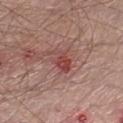Q: Was a biopsy performed?
A: total-body-photography surveillance lesion; no biopsy
Q: Automated lesion metrics?
A: a footprint of about 5 mm² and an eccentricity of roughly 0.85; roughly 9 lightness units darker than nearby skin
Q: Where on the body is the lesion?
A: the right lower leg
Q: Illumination type?
A: white-light illumination
Q: Patient demographics?
A: male, in their mid- to late 50s
Q: How large is the lesion?
A: about 3.5 mm
Q: What kind of image is this?
A: total-body-photography crop, ~15 mm field of view